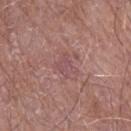Acquisition and patient details:
A male subject aged 68–72. The lesion is on the left upper arm. Cropped from a total-body skin-imaging series; the visible field is about 15 mm. Longest diameter approximately 3 mm. Captured under white-light illumination.